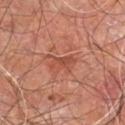Assessment: Recorded during total-body skin imaging; not selected for excision or biopsy. Context: Automated tile analysis of the lesion measured an area of roughly 4.5 mm², an outline eccentricity of about 0.85 (0 = round, 1 = elongated), and a symmetry-axis asymmetry near 0.3. The analysis additionally found an average lesion color of about L≈47 a*≈27 b*≈31 (CIELAB), a lesion–skin lightness drop of about 8, and a normalized border contrast of about 6. And it measured a nevus-likeness score of about 0/100 and a lesion-detection confidence of about 100/100. A male subject, in their 60s. This is a cross-polarized tile. Cropped from a whole-body photographic skin survey; the tile spans about 15 mm. The lesion is on the leg. About 3.5 mm across.